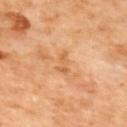Assessment:
Recorded during total-body skin imaging; not selected for excision or biopsy.
Clinical summary:
This image is a 15 mm lesion crop taken from a total-body photograph. An algorithmic analysis of the crop reported an area of roughly 3 mm² and two-axis asymmetry of about 0.4. And it measured a lesion color around L≈64 a*≈25 b*≈44 in CIELAB, about 8 CIELAB-L* units darker than the surrounding skin, and a normalized lesion–skin contrast near 5.5. Located on the upper back. The lesion's longest dimension is about 3 mm. This is a cross-polarized tile.